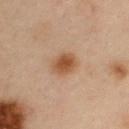Assessment:
No biopsy was performed on this lesion — it was imaged during a full skin examination and was not determined to be concerning.
Background:
Longest diameter approximately 3 mm. The subject is a male in their mid-50s. On the right upper arm. A close-up tile cropped from a whole-body skin photograph, about 15 mm across.